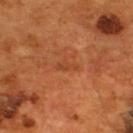Part of a total-body skin-imaging series; this lesion was reviewed on a skin check and was not flagged for biopsy. The patient is a male approximately 55 years of age. Imaged with cross-polarized lighting. This image is a 15 mm lesion crop taken from a total-body photograph. Located on the back. The lesion's longest dimension is about 2.5 mm.